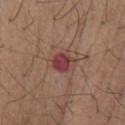Imaged during a routine full-body skin examination; the lesion was not biopsied and no histopathology is available.
From the chest.
A male subject, about 65 years old.
Captured under white-light illumination.
Automated image analysis of the tile measured a lesion area of about 5.5 mm², an outline eccentricity of about 0.5 (0 = round, 1 = elongated), and a shape-asymmetry score of about 0.2 (0 = symmetric). The analysis additionally found about 11 CIELAB-L* units darker than the surrounding skin and a normalized border contrast of about 9.5. The software also gave a classifier nevus-likeness of about 0/100.
A close-up tile cropped from a whole-body skin photograph, about 15 mm across.
Approximately 2.5 mm at its widest.